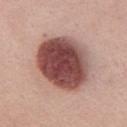Q: Automated lesion metrics?
A: a nevus-likeness score of about 95/100
Q: Where on the body is the lesion?
A: the upper back
Q: What is the lesion's diameter?
A: about 8 mm
Q: What are the patient's age and sex?
A: female, in their mid-30s
Q: Illumination type?
A: white-light
Q: How was this image acquired?
A: total-body-photography crop, ~15 mm field of view